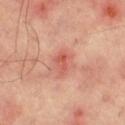  image:
    source: total-body photography crop
    field_of_view_mm: 15
  lesion_size:
    long_diameter_mm_approx: 2.5
  automated_metrics:
    area_mm2_approx: 3.5
    eccentricity: 0.8
    shape_asymmetry: 0.3
  site: right thigh